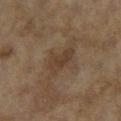workup = imaged on a skin check; not biopsied | image source = total-body-photography crop, ~15 mm field of view | body site = the left lower leg | patient = female, about 60 years old | diameter = about 4 mm.